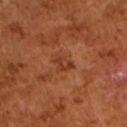The lesion was photographed on a routine skin check and not biopsied; there is no pathology result. Cropped from a whole-body photographic skin survey; the tile spans about 15 mm. Measured at roughly 2.5 mm in maximum diameter. A male subject aged 63 to 67.